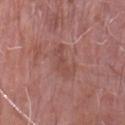Captured during whole-body skin photography for melanoma surveillance; the lesion was not biopsied. A lesion tile, about 15 mm wide, cut from a 3D total-body photograph. About 4 mm across. The subject is a male in their mid-70s. From the right forearm. Captured under white-light illumination.